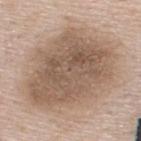follow-up: catalogued during a skin exam; not biopsied | location: the upper back | acquisition: total-body-photography crop, ~15 mm field of view | subject: male, aged 43–47.